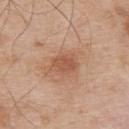Q: Is there a histopathology result?
A: no biopsy performed (imaged during a skin exam)
Q: Automated lesion metrics?
A: a lesion area of about 5 mm² and an eccentricity of roughly 0.75; an average lesion color of about L≈54 a*≈23 b*≈32 (CIELAB), roughly 9 lightness units darker than nearby skin, and a lesion-to-skin contrast of about 6.5 (normalized; higher = more distinct); border irregularity of about 2 on a 0–10 scale and peripheral color asymmetry of about 1; an automated nevus-likeness rating near 5 out of 100 and lesion-presence confidence of about 100/100
Q: What are the patient's age and sex?
A: male, approximately 55 years of age
Q: How was the tile lit?
A: white-light illumination
Q: What kind of image is this?
A: ~15 mm crop, total-body skin-cancer survey
Q: How large is the lesion?
A: about 3 mm
Q: What is the anatomic site?
A: the upper back